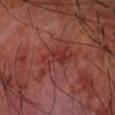Impression:
Imaged during a routine full-body skin examination; the lesion was not biopsied and no histopathology is available.
Clinical summary:
On the right forearm. Automated image analysis of the tile measured an area of roughly 11 mm², an outline eccentricity of about 0.85 (0 = round, 1 = elongated), and a symmetry-axis asymmetry near 0.4. And it measured roughly 6 lightness units darker than nearby skin and a normalized border contrast of about 6. A male subject aged 68 to 72. Longest diameter approximately 5.5 mm. A lesion tile, about 15 mm wide, cut from a 3D total-body photograph. The tile uses cross-polarized illumination.